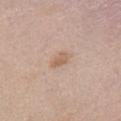Background:
A female subject, roughly 40 years of age. About 2.5 mm across. This image is a 15 mm lesion crop taken from a total-body photograph. The tile uses white-light illumination. The lesion is located on the abdomen. Automated image analysis of the tile measured border irregularity of about 2.5 on a 0–10 scale, internal color variation of about 1.5 on a 0–10 scale, and a peripheral color-asymmetry measure near 0.5. The analysis additionally found a classifier nevus-likeness of about 35/100.